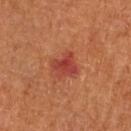This lesion was catalogued during total-body skin photography and was not selected for biopsy. The lesion is on the left arm. A female patient aged around 65. Approximately 3 mm at its widest. This is a cross-polarized tile. The total-body-photography lesion software estimated an outline eccentricity of about 0.4 (0 = round, 1 = elongated) and a shape-asymmetry score of about 0.35 (0 = symmetric). The software also gave internal color variation of about 4 on a 0–10 scale. It also reported an automated nevus-likeness rating near 5 out of 100 and a lesion-detection confidence of about 100/100. This image is a 15 mm lesion crop taken from a total-body photograph.